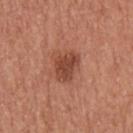Clinical impression: Recorded during total-body skin imaging; not selected for excision or biopsy. Image and clinical context: The subject is a male in their mid- to late 60s. On the right upper arm. The total-body-photography lesion software estimated about 11 CIELAB-L* units darker than the surrounding skin and a lesion-to-skin contrast of about 8 (normalized; higher = more distinct). The software also gave radial color variation of about 1. And it measured a nevus-likeness score of about 75/100 and a lesion-detection confidence of about 100/100. Imaged with white-light lighting. About 3.5 mm across. Cropped from a whole-body photographic skin survey; the tile spans about 15 mm.